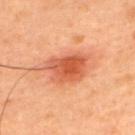follow-up: no biopsy performed (imaged during a skin exam)
body site: the upper back
subject: male, roughly 45 years of age
automated metrics: a footprint of about 15 mm², an outline eccentricity of about 0.75 (0 = round, 1 = elongated), and a shape-asymmetry score of about 0.25 (0 = symmetric); a mean CIELAB color near L≈56 a*≈31 b*≈37, about 13 CIELAB-L* units darker than the surrounding skin, and a lesion-to-skin contrast of about 8 (normalized; higher = more distinct); lesion-presence confidence of about 100/100
imaging modality: 15 mm crop, total-body photography
lighting: cross-polarized illumination
lesion size: ≈6 mm